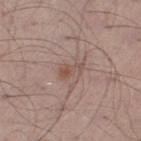biopsy status = total-body-photography surveillance lesion; no biopsy | body site = the right thigh | lighting = white-light illumination | subject = male, in their mid-50s | lesion diameter = ~3 mm (longest diameter) | acquisition = total-body-photography crop, ~15 mm field of view.